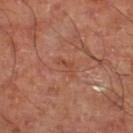{"biopsy_status": "not biopsied; imaged during a skin examination", "patient": {"sex": "male", "age_approx": 65}, "image": {"source": "total-body photography crop", "field_of_view_mm": 15}, "site": "right lower leg", "lesion_size": {"long_diameter_mm_approx": 2.5}}